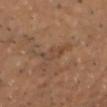The lesion was tiled from a total-body skin photograph and was not biopsied.
A 15 mm close-up tile from a total-body photography series done for melanoma screening.
From the head or neck.
The subject is a male approximately 45 years of age.